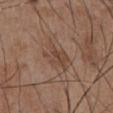Q: Is there a histopathology result?
A: imaged on a skin check; not biopsied
Q: What did automated image analysis measure?
A: an average lesion color of about L≈45 a*≈18 b*≈27 (CIELAB), roughly 7 lightness units darker than nearby skin, and a lesion-to-skin contrast of about 6 (normalized; higher = more distinct)
Q: Where on the body is the lesion?
A: the abdomen
Q: How was the tile lit?
A: white-light
Q: What are the patient's age and sex?
A: male, aged 53–57
Q: What kind of image is this?
A: ~15 mm crop, total-body skin-cancer survey